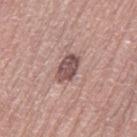biopsy_status: not biopsied; imaged during a skin examination
image:
  source: total-body photography crop
  field_of_view_mm: 15
lighting: white-light
patient:
  sex: female
  age_approx: 85+
automated_metrics:
  area_mm2_approx: 6.0
  eccentricity: 0.8
  cielab_L: 51
  cielab_a: 19
  cielab_b: 20
  vs_skin_darker_L: 14.0
  vs_skin_contrast_norm: 9.5
site: left thigh
lesion_size:
  long_diameter_mm_approx: 3.5